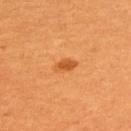The lesion was photographed on a routine skin check and not biopsied; there is no pathology result.
The recorded lesion diameter is about 2.5 mm.
Automated tile analysis of the lesion measured an average lesion color of about L≈56 a*≈31 b*≈49 (CIELAB), about 10 CIELAB-L* units darker than the surrounding skin, and a normalized border contrast of about 7. The software also gave a within-lesion color-variation index near 1.5/10 and radial color variation of about 0.5. The analysis additionally found an automated nevus-likeness rating near 95 out of 100 and a lesion-detection confidence of about 100/100.
The tile uses cross-polarized illumination.
A close-up tile cropped from a whole-body skin photograph, about 15 mm across.
The subject is a female approximately 50 years of age.